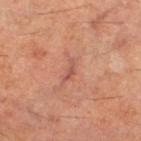* workup: total-body-photography surveillance lesion; no biopsy
* tile lighting: cross-polarized
* image source: 15 mm crop, total-body photography
* body site: the left lower leg
* subject: male, aged 58 to 62